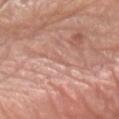The lesion was photographed on a routine skin check and not biopsied; there is no pathology result.
The lesion's longest dimension is about 1.5 mm.
A female patient aged 48 to 52.
Imaged with white-light lighting.
Automated tile analysis of the lesion measured a footprint of about 1 mm² and a shape-asymmetry score of about 0.3 (0 = symmetric). The software also gave a border-irregularity index near 2.5/10, a color-variation rating of about 0/10, and peripheral color asymmetry of about 0.
A 15 mm close-up extracted from a 3D total-body photography capture.
On the left forearm.